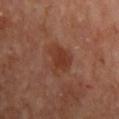Impression:
This lesion was catalogued during total-body skin photography and was not selected for biopsy.
Clinical summary:
The recorded lesion diameter is about 4.5 mm. A 15 mm crop from a total-body photograph taken for skin-cancer surveillance. A male subject approximately 70 years of age. Automated image analysis of the tile measured an area of roughly 9.5 mm² and two-axis asymmetry of about 0.35. It also reported roughly 7 lightness units darker than nearby skin. The software also gave an automated nevus-likeness rating near 40 out of 100 and lesion-presence confidence of about 100/100. The lesion is on the mid back. This is a cross-polarized tile.